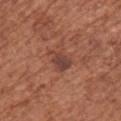{
  "biopsy_status": "not biopsied; imaged during a skin examination",
  "site": "back",
  "image": {
    "source": "total-body photography crop",
    "field_of_view_mm": 15
  },
  "lesion_size": {
    "long_diameter_mm_approx": 3.5
  },
  "patient": {
    "sex": "female",
    "age_approx": 75
  },
  "automated_metrics": {
    "cielab_L": 43,
    "cielab_a": 23,
    "cielab_b": 26,
    "vs_skin_darker_L": 8.0,
    "vs_skin_contrast_norm": 7.5,
    "nevus_likeness_0_100": 20
  },
  "lighting": "white-light"
}